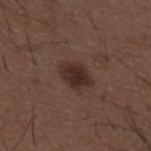biopsy status = imaged on a skin check; not biopsied.